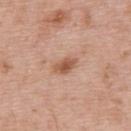Impression:
Recorded during total-body skin imaging; not selected for excision or biopsy.
Image and clinical context:
The lesion is on the upper back. Longest diameter approximately 3 mm. A male patient aged 63–67. The total-body-photography lesion software estimated a lesion area of about 4 mm², an eccentricity of roughly 0.8, and a symmetry-axis asymmetry near 0.25. It also reported a border-irregularity index near 2.5/10, a within-lesion color-variation index near 3.5/10, and a peripheral color-asymmetry measure near 1. And it measured an automated nevus-likeness rating near 85 out of 100. Imaged with white-light lighting. This image is a 15 mm lesion crop taken from a total-body photograph.